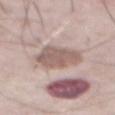The lesion was tiled from a total-body skin photograph and was not biopsied.
The tile uses white-light illumination.
About 4.5 mm across.
A 15 mm crop from a total-body photograph taken for skin-cancer surveillance.
A male subject aged around 75.
From the abdomen.